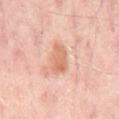| feature | finding |
|---|---|
| follow-up | catalogued during a skin exam; not biopsied |
| image | 15 mm crop, total-body photography |
| lighting | cross-polarized |
| patient | about 55 years old |
| site | the back |
| size | ≈3.5 mm |